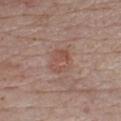{
  "biopsy_status": "not biopsied; imaged during a skin examination",
  "automated_metrics": {
    "area_mm2_approx": 8.0,
    "cielab_L": 51,
    "cielab_a": 20,
    "cielab_b": 25,
    "vs_skin_contrast_norm": 5.0,
    "border_irregularity_0_10": 2.0,
    "color_variation_0_10": 4.0,
    "peripheral_color_asymmetry": 1.5
  },
  "lesion_size": {
    "long_diameter_mm_approx": 3.5
  },
  "patient": {
    "sex": "male",
    "age_approx": 75
  },
  "lighting": "white-light",
  "image": {
    "source": "total-body photography crop",
    "field_of_view_mm": 15
  },
  "site": "chest"
}